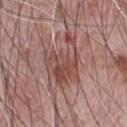workup: catalogued during a skin exam; not biopsied
acquisition: 15 mm crop, total-body photography
subject: male, aged approximately 70
size: about 7 mm
image-analysis metrics: an area of roughly 19 mm², a shape eccentricity near 0.7, and a shape-asymmetry score of about 0.45 (0 = symmetric); a lesion color around L≈48 a*≈22 b*≈23 in CIELAB and a normalized border contrast of about 7; a border-irregularity rating of about 6.5/10, internal color variation of about 6 on a 0–10 scale, and radial color variation of about 2; a classifier nevus-likeness of about 0/100 and a lesion-detection confidence of about 100/100
location: the chest